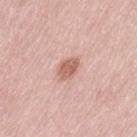Notes:
- notes · total-body-photography surveillance lesion; no biopsy
- tile lighting · white-light illumination
- diameter · ≈3 mm
- subject · female, roughly 65 years of age
- image · 15 mm crop, total-body photography
- anatomic site · the leg